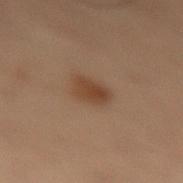Findings:
- workup · total-body-photography surveillance lesion; no biopsy
- image source · 15 mm crop, total-body photography
- site · the lower back
- subject · male, roughly 55 years of age